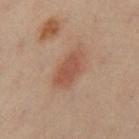Impression:
The lesion was photographed on a routine skin check and not biopsied; there is no pathology result.
Acquisition and patient details:
A 15 mm crop from a total-body photograph taken for skin-cancer surveillance. A female patient aged around 40. The lesion-visualizer software estimated a footprint of about 8.5 mm², an outline eccentricity of about 0.9 (0 = round, 1 = elongated), and two-axis asymmetry of about 0.2. And it measured an average lesion color of about L≈52 a*≈22 b*≈30 (CIELAB) and roughly 9 lightness units darker than nearby skin. This is a cross-polarized tile. From the left leg.